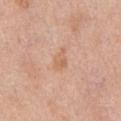Clinical impression: Captured during whole-body skin photography for melanoma surveillance; the lesion was not biopsied. Image and clinical context: The lesion is on the abdomen. Imaged with white-light lighting. A male patient approximately 70 years of age. The total-body-photography lesion software estimated a footprint of about 4 mm² and an eccentricity of roughly 0.85. And it measured a lesion color around L≈63 a*≈21 b*≈33 in CIELAB, roughly 7 lightness units darker than nearby skin, and a normalized border contrast of about 5.5. And it measured a nevus-likeness score of about 0/100 and lesion-presence confidence of about 100/100. The lesion's longest dimension is about 3 mm. A 15 mm close-up tile from a total-body photography series done for melanoma screening.